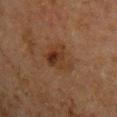<record>
  <biopsy_status>not biopsied; imaged during a skin examination</biopsy_status>
  <automated_metrics>
    <area_mm2_approx>9.5</area_mm2_approx>
    <eccentricity>0.6</eccentricity>
    <shape_asymmetry>0.45</shape_asymmetry>
    <vs_skin_contrast_norm>7.5</vs_skin_contrast_norm>
    <peripheral_color_asymmetry>2.5</peripheral_color_asymmetry>
    <nevus_likeness_0_100>75</nevus_likeness_0_100>
    <lesion_detection_confidence_0_100>100</lesion_detection_confidence_0_100>
  </automated_metrics>
  <site>chest</site>
  <lighting>cross-polarized</lighting>
  <image>
    <source>total-body photography crop</source>
    <field_of_view_mm>15</field_of_view_mm>
  </image>
  <lesion_size>
    <long_diameter_mm_approx>4.0</long_diameter_mm_approx>
  </lesion_size>
  <patient>
    <sex>male</sex>
    <age_approx>50</age_approx>
  </patient>
</record>